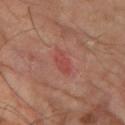<case>
  <biopsy_status>not biopsied; imaged during a skin examination</biopsy_status>
  <patient>
    <sex>male</sex>
    <age_approx>65</age_approx>
  </patient>
  <image>
    <source>total-body photography crop</source>
    <field_of_view_mm>15</field_of_view_mm>
  </image>
  <site>left thigh</site>
  <lesion_size>
    <long_diameter_mm_approx>3.0</long_diameter_mm_approx>
  </lesion_size>
</case>